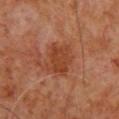notes: catalogued during a skin exam; not biopsied | anatomic site: the upper back | imaging modality: ~15 mm tile from a whole-body skin photo | patient: male, roughly 60 years of age | automated lesion analysis: a border-irregularity index near 3/10 | tile lighting: cross-polarized illumination.